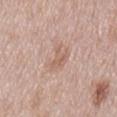The lesion was photographed on a routine skin check and not biopsied; there is no pathology result. A roughly 15 mm field-of-view crop from a total-body skin photograph. Longest diameter approximately 4 mm. The lesion is located on the mid back. This is a white-light tile. A male subject, aged approximately 55.